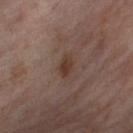Recorded during total-body skin imaging; not selected for excision or biopsy. A female patient, aged 53 to 57. A 15 mm close-up tile from a total-body photography series done for melanoma screening. On the leg. Approximately 2.5 mm at its widest.